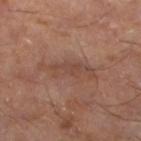Impression: Part of a total-body skin-imaging series; this lesion was reviewed on a skin check and was not flagged for biopsy. Image and clinical context: A lesion tile, about 15 mm wide, cut from a 3D total-body photograph. On the left lower leg. About 6.5 mm across. Automated tile analysis of the lesion measured a lesion area of about 11 mm², an outline eccentricity of about 0.95 (0 = round, 1 = elongated), and a symmetry-axis asymmetry near 0.3. The software also gave border irregularity of about 5 on a 0–10 scale, internal color variation of about 3 on a 0–10 scale, and a peripheral color-asymmetry measure near 1. The software also gave a classifier nevus-likeness of about 0/100 and a lesion-detection confidence of about 95/100. The patient is a male aged approximately 55.